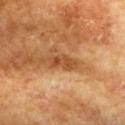A region of skin cropped from a whole-body photographic capture, roughly 15 mm wide. The subject is a female approximately 75 years of age. Automated image analysis of the tile measured an area of roughly 5 mm² and an outline eccentricity of about 0.9 (0 = round, 1 = elongated). It also reported an average lesion color of about L≈51 a*≈26 b*≈41 (CIELAB), a lesion–skin lightness drop of about 11, and a normalized border contrast of about 7.5. And it measured border irregularity of about 3 on a 0–10 scale, a color-variation rating of about 4.5/10, and radial color variation of about 1.5. The software also gave a classifier nevus-likeness of about 0/100 and a detector confidence of about 85 out of 100 that the crop contains a lesion. The lesion is on the chest.